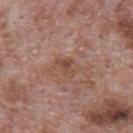A male subject in their 70s. The lesion is on the mid back. The tile uses white-light illumination. The total-body-photography lesion software estimated a footprint of about 3 mm², a shape eccentricity near 0.8, and two-axis asymmetry of about 0.45. The analysis additionally found an average lesion color of about L≈47 a*≈21 b*≈28 (CIELAB), about 8 CIELAB-L* units darker than the surrounding skin, and a normalized border contrast of about 6. The software also gave an automated nevus-likeness rating near 0 out of 100. Cropped from a whole-body photographic skin survey; the tile spans about 15 mm.